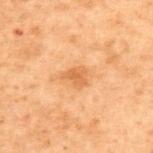Recorded during total-body skin imaging; not selected for excision or biopsy.